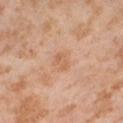The lesion was photographed on a routine skin check and not biopsied; there is no pathology result.
A roughly 15 mm field-of-view crop from a total-body skin photograph.
Longest diameter approximately 2.5 mm.
Imaged with cross-polarized lighting.
The patient is a female aged around 55.
Located on the right thigh.